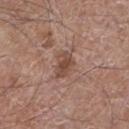Recorded during total-body skin imaging; not selected for excision or biopsy. The lesion-visualizer software estimated two-axis asymmetry of about 0.2. The analysis additionally found a mean CIELAB color near L≈47 a*≈20 b*≈26 and about 10 CIELAB-L* units darker than the surrounding skin. The patient is a male aged around 60. A lesion tile, about 15 mm wide, cut from a 3D total-body photograph. Located on the left lower leg. Longest diameter approximately 3 mm.